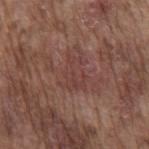Background: From the mid back. About 4 mm across. Cropped from a total-body skin-imaging series; the visible field is about 15 mm. A male subject, roughly 75 years of age. The tile uses white-light illumination.